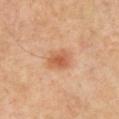Impression: This lesion was catalogued during total-body skin photography and was not selected for biopsy. Clinical summary: A male patient aged around 55. The tile uses cross-polarized illumination. Located on the chest. A 15 mm close-up tile from a total-body photography series done for melanoma screening. The lesion's longest dimension is about 3 mm.